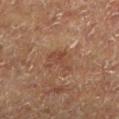notes=total-body-photography surveillance lesion; no biopsy | TBP lesion metrics=a mean CIELAB color near L≈35 a*≈17 b*≈24, about 6 CIELAB-L* units darker than the surrounding skin, and a normalized lesion–skin contrast near 5.5; an automated nevus-likeness rating near 0 out of 100 and a lesion-detection confidence of about 100/100 | patient=male, aged around 75 | image=~15 mm crop, total-body skin-cancer survey | location=the left lower leg | illumination=cross-polarized illumination | lesion size=about 4 mm.